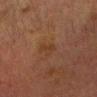Assessment:
The lesion was photographed on a routine skin check and not biopsied; there is no pathology result.